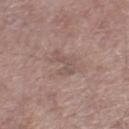workup = catalogued during a skin exam; not biopsied | image = 15 mm crop, total-body photography | diameter = about 4 mm | patient = male, about 70 years old | site = the right thigh | illumination = white-light illumination.